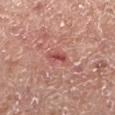  biopsy_status: not biopsied; imaged during a skin examination
  patient:
    sex: male
    age_approx: 55
  lesion_size:
    long_diameter_mm_approx: 2.5
  site: left lower leg
  automated_metrics:
    area_mm2_approx: 3.0
    eccentricity: 0.9
    shape_asymmetry: 0.3
  lighting: white-light
  image:
    source: total-body photography crop
    field_of_view_mm: 15